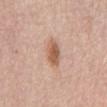Q: What is the anatomic site?
A: the abdomen
Q: Automated lesion metrics?
A: a footprint of about 6 mm², an eccentricity of roughly 0.8, and two-axis asymmetry of about 0.15
Q: What kind of image is this?
A: ~15 mm tile from a whole-body skin photo
Q: Who is the patient?
A: female, in their mid- to late 60s
Q: How was the tile lit?
A: white-light illumination